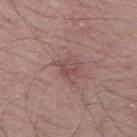Notes:
* workup · imaged on a skin check; not biopsied
* lesion diameter · about 3 mm
* lighting · white-light illumination
* patient · male, aged approximately 50
* automated lesion analysis · an automated nevus-likeness rating near 0 out of 100
* site · the left thigh
* acquisition · ~15 mm tile from a whole-body skin photo